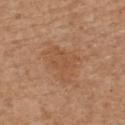Impression: Captured during whole-body skin photography for melanoma surveillance; the lesion was not biopsied. Background: The recorded lesion diameter is about 5 mm. Imaged with white-light lighting. The total-body-photography lesion software estimated a lesion area of about 12 mm², an outline eccentricity of about 0.7 (0 = round, 1 = elongated), and a shape-asymmetry score of about 0.4 (0 = symmetric). And it measured border irregularity of about 4.5 on a 0–10 scale and peripheral color asymmetry of about 0.5. The lesion is located on the upper back. A male patient about 70 years old. A region of skin cropped from a whole-body photographic capture, roughly 15 mm wide.